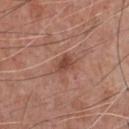<record>
<biopsy_status>not biopsied; imaged during a skin examination</biopsy_status>
<lesion_size>
  <long_diameter_mm_approx>2.5</long_diameter_mm_approx>
</lesion_size>
<patient>
  <sex>male</sex>
  <age_approx>70</age_approx>
</patient>
<site>chest</site>
<lighting>white-light</lighting>
<image>
  <source>total-body photography crop</source>
  <field_of_view_mm>15</field_of_view_mm>
</image>
</record>